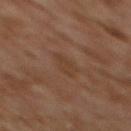Clinical impression:
The lesion was photographed on a routine skin check and not biopsied; there is no pathology result.
Background:
The lesion's longest dimension is about 3 mm. From the upper back. Cropped from a whole-body photographic skin survey; the tile spans about 15 mm. An algorithmic analysis of the crop reported a border-irregularity index near 3/10, a color-variation rating of about 1/10, and radial color variation of about 0.5. The subject is a female about 60 years old.